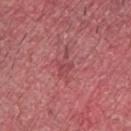notes = imaged on a skin check; not biopsied | patient = male, about 40 years old | body site = the arm | image source = ~15 mm tile from a whole-body skin photo.